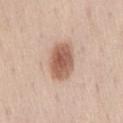Assessment:
This lesion was catalogued during total-body skin photography and was not selected for biopsy.
Acquisition and patient details:
The lesion is on the back. A 15 mm close-up extracted from a 3D total-body photography capture. A male subject, roughly 75 years of age.